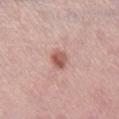biopsy status: catalogued during a skin exam; not biopsied
anatomic site: the right lower leg
lesion diameter: ~2.5 mm (longest diameter)
automated lesion analysis: a footprint of about 4 mm², an eccentricity of roughly 0.6, and a symmetry-axis asymmetry near 0.15; peripheral color asymmetry of about 1.5
tile lighting: white-light
subject: female, aged approximately 45
imaging modality: total-body-photography crop, ~15 mm field of view